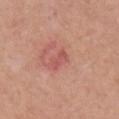Assessment: This lesion was catalogued during total-body skin photography and was not selected for biopsy. Image and clinical context: A roughly 15 mm field-of-view crop from a total-body skin photograph. A male patient, approximately 55 years of age. Located on the front of the torso.